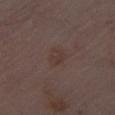Findings:
• location — the left lower leg
• image — 15 mm crop, total-body photography
• subject — male, about 70 years old